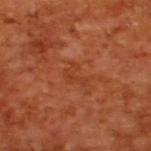The lesion was photographed on a routine skin check and not biopsied; there is no pathology result. Measured at roughly 3 mm in maximum diameter. A male patient roughly 65 years of age. A roughly 15 mm field-of-view crop from a total-body skin photograph. This is a cross-polarized tile. Automated tile analysis of the lesion measured a nevus-likeness score of about 0/100 and a detector confidence of about 100 out of 100 that the crop contains a lesion.